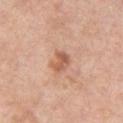No biopsy was performed on this lesion — it was imaged during a full skin examination and was not determined to be concerning.
A roughly 15 mm field-of-view crop from a total-body skin photograph.
The patient is a male approximately 60 years of age.
The lesion is located on the chest.
Imaged with white-light lighting.
The recorded lesion diameter is about 2.5 mm.